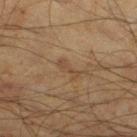Notes:
– notes · total-body-photography surveillance lesion; no biopsy
– size · ≈3 mm
– location · the right thigh
– acquisition · ~15 mm tile from a whole-body skin photo
– lighting · cross-polarized
– patient · male, about 60 years old